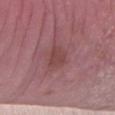{
  "biopsy_status": "not biopsied; imaged during a skin examination",
  "patient": {
    "sex": "male",
    "age_approx": 65
  },
  "lighting": "white-light",
  "lesion_size": {
    "long_diameter_mm_approx": 3.5
  },
  "image": {
    "source": "total-body photography crop",
    "field_of_view_mm": 15
  },
  "site": "right forearm"
}